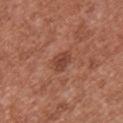Case summary:
• workup — imaged on a skin check; not biopsied
• body site — the chest
• lesion diameter — about 2.5 mm
• TBP lesion metrics — a lesion area of about 4 mm², an outline eccentricity of about 0.65 (0 = round, 1 = elongated), and a symmetry-axis asymmetry near 0.3; a lesion color around L≈43 a*≈25 b*≈29 in CIELAB, roughly 9 lightness units darker than nearby skin, and a lesion-to-skin contrast of about 7 (normalized; higher = more distinct); a border-irregularity rating of about 2.5/10, a color-variation rating of about 2/10, and radial color variation of about 0.5
• image — total-body-photography crop, ~15 mm field of view
• subject — female, aged around 40